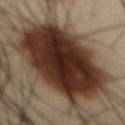Q: Is there a histopathology result?
A: no biopsy performed (imaged during a skin exam)
Q: How was this image acquired?
A: 15 mm crop, total-body photography
Q: Lesion location?
A: the abdomen
Q: Automated lesion metrics?
A: roughly 17 lightness units darker than nearby skin
Q: Patient demographics?
A: male, aged 33–37
Q: Illumination type?
A: cross-polarized illumination